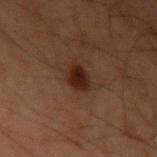Part of a total-body skin-imaging series; this lesion was reviewed on a skin check and was not flagged for biopsy.
The patient is a male aged around 60.
The lesion-visualizer software estimated an average lesion color of about L≈17 a*≈16 b*≈19 (CIELAB), roughly 9 lightness units darker than nearby skin, and a normalized lesion–skin contrast near 11.
Cropped from a whole-body photographic skin survey; the tile spans about 15 mm.
Longest diameter approximately 2.5 mm.
From the left upper arm.